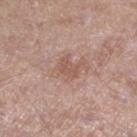notes: imaged on a skin check; not biopsied
size: ≈2.5 mm
acquisition: 15 mm crop, total-body photography
patient: male, aged 43 to 47
location: the leg
automated metrics: a lesion color around L≈54 a*≈20 b*≈26 in CIELAB, about 7 CIELAB-L* units darker than the surrounding skin, and a normalized border contrast of about 5.5; border irregularity of about 3.5 on a 0–10 scale and a within-lesion color-variation index near 1/10; a nevus-likeness score of about 0/100 and a lesion-detection confidence of about 100/100